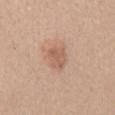biopsy_status: not biopsied; imaged during a skin examination
lighting: white-light
patient:
  sex: female
  age_approx: 45
automated_metrics:
  eccentricity: 0.7
  shape_asymmetry: 0.25
  vs_skin_contrast_norm: 6.0
lesion_size:
  long_diameter_mm_approx: 3.0
image:
  source: total-body photography crop
  field_of_view_mm: 15
site: back